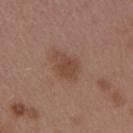<tbp_lesion>
  <biopsy_status>not biopsied; imaged during a skin examination</biopsy_status>
  <lighting>white-light</lighting>
  <image>
    <source>total-body photography crop</source>
    <field_of_view_mm>15</field_of_view_mm>
  </image>
  <automated_metrics>
    <area_mm2_approx>6.0</area_mm2_approx>
    <shape_asymmetry>0.25</shape_asymmetry>
    <border_irregularity_0_10>2.5</border_irregularity_0_10>
    <color_variation_0_10>1.5</color_variation_0_10>
    <peripheral_color_asymmetry>0.5</peripheral_color_asymmetry>
    <nevus_likeness_0_100>70</nevus_likeness_0_100>
    <lesion_detection_confidence_0_100>100</lesion_detection_confidence_0_100>
  </automated_metrics>
  <patient>
    <sex>female</sex>
    <age_approx>45</age_approx>
  </patient>
  <site>right upper arm</site>
</tbp_lesion>